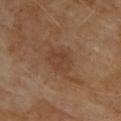{
  "biopsy_status": "not biopsied; imaged during a skin examination",
  "lesion_size": {
    "long_diameter_mm_approx": 3.5
  },
  "patient": {
    "sex": "male",
    "age_approx": 70
  },
  "lighting": "cross-polarized",
  "image": {
    "source": "total-body photography crop",
    "field_of_view_mm": 15
  },
  "site": "upper back"
}